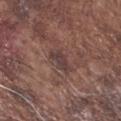Impression:
This lesion was catalogued during total-body skin photography and was not selected for biopsy.
Clinical summary:
A male subject, aged around 75. A lesion tile, about 15 mm wide, cut from a 3D total-body photograph. On the right forearm.